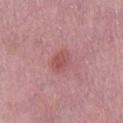tile lighting = white-light
location = the right lower leg
imaging modality = ~15 mm crop, total-body skin-cancer survey
lesion diameter = about 3 mm
subject = female, aged around 60